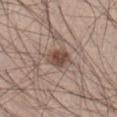No biopsy was performed on this lesion — it was imaged during a full skin examination and was not determined to be concerning.
Imaged with white-light lighting.
A male subject approximately 65 years of age.
Longest diameter approximately 3 mm.
From the front of the torso.
A 15 mm close-up extracted from a 3D total-body photography capture.